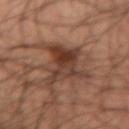notes = total-body-photography surveillance lesion; no biopsy | size = ~7 mm (longest diameter) | patient = male, aged around 35 | site = the left forearm | acquisition = ~15 mm tile from a whole-body skin photo | tile lighting = cross-polarized.